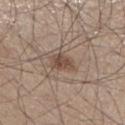Clinical impression: This lesion was catalogued during total-body skin photography and was not selected for biopsy. Acquisition and patient details: The lesion-visualizer software estimated a lesion area of about 4.5 mm² and a symmetry-axis asymmetry near 0.3. It also reported an average lesion color of about L≈47 a*≈16 b*≈25 (CIELAB) and a normalized border contrast of about 8. And it measured a border-irregularity rating of about 2.5/10 and radial color variation of about 0.5. Imaged with white-light lighting. A male patient, about 45 years old. On the right lower leg. Cropped from a total-body skin-imaging series; the visible field is about 15 mm.